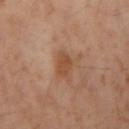Q: Is there a histopathology result?
A: total-body-photography surveillance lesion; no biopsy
Q: What did automated image analysis measure?
A: a lesion color around L≈49 a*≈22 b*≈33 in CIELAB and a lesion-to-skin contrast of about 7 (normalized; higher = more distinct); border irregularity of about 2 on a 0–10 scale, a within-lesion color-variation index near 2/10, and peripheral color asymmetry of about 0.5
Q: What is the imaging modality?
A: 15 mm crop, total-body photography
Q: How was the tile lit?
A: cross-polarized
Q: Patient demographics?
A: female, roughly 45 years of age
Q: Lesion location?
A: the arm
Q: How large is the lesion?
A: about 3 mm